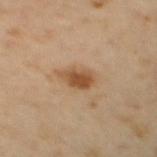Findings:
* biopsy status: total-body-photography surveillance lesion; no biopsy
* imaging modality: ~15 mm tile from a whole-body skin photo
* subject: female, aged 53 to 57
* location: the back